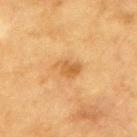workup: total-body-photography surveillance lesion; no biopsy | patient: male, aged 83 or older | illumination: cross-polarized illumination | lesion size: ~3.5 mm (longest diameter) | automated metrics: a lesion area of about 5 mm², an eccentricity of roughly 0.75, and two-axis asymmetry of about 0.3; a lesion color around L≈53 a*≈20 b*≈41 in CIELAB, a lesion–skin lightness drop of about 9, and a normalized border contrast of about 6.5; an automated nevus-likeness rating near 0 out of 100 and a detector confidence of about 100 out of 100 that the crop contains a lesion | image source: total-body-photography crop, ~15 mm field of view | site: the upper back.